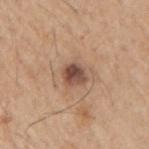<case>
<biopsy_status>not biopsied; imaged during a skin examination</biopsy_status>
<site>right upper arm</site>
<image>
  <source>total-body photography crop</source>
  <field_of_view_mm>15</field_of_view_mm>
</image>
<patient>
  <sex>male</sex>
  <age_approx>65</age_approx>
</patient>
</case>